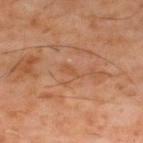{
  "biopsy_status": "not biopsied; imaged during a skin examination",
  "automated_metrics": {
    "area_mm2_approx": 2.5,
    "eccentricity": 0.95,
    "shape_asymmetry": 0.35,
    "cielab_L": 49,
    "cielab_a": 22,
    "cielab_b": 34,
    "vs_skin_contrast_norm": 4.5,
    "lesion_detection_confidence_0_100": 95
  },
  "patient": {
    "sex": "male",
    "age_approx": 60
  },
  "lighting": "cross-polarized",
  "image": {
    "source": "total-body photography crop",
    "field_of_view_mm": 15
  },
  "site": "mid back",
  "lesion_size": {
    "long_diameter_mm_approx": 3.0
  }
}